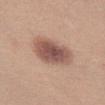| feature | finding |
|---|---|
| follow-up | catalogued during a skin exam; not biopsied |
| tile lighting | white-light |
| patient | female, aged 18–22 |
| anatomic site | the left thigh |
| diameter | ~5 mm (longest diameter) |
| acquisition | total-body-photography crop, ~15 mm field of view |